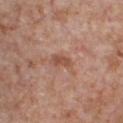No biopsy was performed on this lesion — it was imaged during a full skin examination and was not determined to be concerning. An algorithmic analysis of the crop reported border irregularity of about 3 on a 0–10 scale, a color-variation rating of about 1.5/10, and a peripheral color-asymmetry measure near 0.5. The analysis additionally found an automated nevus-likeness rating near 0 out of 100 and lesion-presence confidence of about 100/100. On the chest. The subject is a male aged approximately 65. Longest diameter approximately 2.5 mm. A 15 mm close-up extracted from a 3D total-body photography capture.